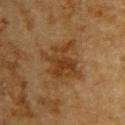Captured during whole-body skin photography for melanoma surveillance; the lesion was not biopsied.
A lesion tile, about 15 mm wide, cut from a 3D total-body photograph.
The lesion is located on the upper back.
An algorithmic analysis of the crop reported a footprint of about 14 mm², a shape eccentricity near 0.45, and two-axis asymmetry of about 0.4. It also reported a border-irregularity rating of about 6.5/10, internal color variation of about 4 on a 0–10 scale, and radial color variation of about 1.5. The software also gave an automated nevus-likeness rating near 20 out of 100 and a detector confidence of about 100 out of 100 that the crop contains a lesion.
Captured under cross-polarized illumination.
A male patient, aged 83 or over.
Longest diameter approximately 4.5 mm.